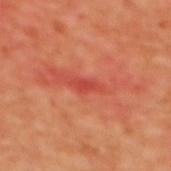biopsy_status: not biopsied; imaged during a skin examination
site: upper back
image:
  source: total-body photography crop
  field_of_view_mm: 15
patient:
  sex: female
  age_approx: 40